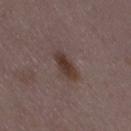notes — no biopsy performed (imaged during a skin exam) | image — 15 mm crop, total-body photography | patient — female, aged 33 to 37 | anatomic site — the right thigh.